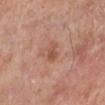notes = no biopsy performed (imaged during a skin exam) | location = the left lower leg | acquisition = total-body-photography crop, ~15 mm field of view | subject = male, approximately 80 years of age.